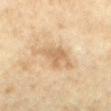Q: Is there a histopathology result?
A: total-body-photography surveillance lesion; no biopsy
Q: Where on the body is the lesion?
A: the back
Q: What is the lesion's diameter?
A: ≈3 mm
Q: What is the imaging modality?
A: total-body-photography crop, ~15 mm field of view
Q: Who is the patient?
A: female, aged 58 to 62